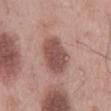The lesion was tiled from a total-body skin photograph and was not biopsied. Measured at roughly 5.5 mm in maximum diameter. The total-body-photography lesion software estimated a lesion color around L≈51 a*≈22 b*≈23 in CIELAB and about 13 CIELAB-L* units darker than the surrounding skin. The software also gave border irregularity of about 2 on a 0–10 scale, a within-lesion color-variation index near 3/10, and peripheral color asymmetry of about 1. From the back. A 15 mm crop from a total-body photograph taken for skin-cancer surveillance. A male patient roughly 55 years of age. Imaged with white-light lighting.